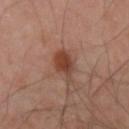Imaged during a routine full-body skin examination; the lesion was not biopsied and no histopathology is available. The lesion is located on the right forearm. A male patient, aged approximately 45. The recorded lesion diameter is about 3.5 mm. A close-up tile cropped from a whole-body skin photograph, about 15 mm across.